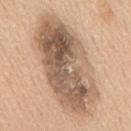Imaged with white-light lighting. Located on the mid back. Cropped from a total-body skin-imaging series; the visible field is about 15 mm. The subject is a male in their 60s. An algorithmic analysis of the crop reported an area of roughly 55 mm², a shape eccentricity near 0.9, and a symmetry-axis asymmetry near 0.1.